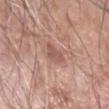Captured during whole-body skin photography for melanoma surveillance; the lesion was not biopsied.
A close-up tile cropped from a whole-body skin photograph, about 15 mm across.
A male patient about 60 years old.
On the right forearm.
An algorithmic analysis of the crop reported an average lesion color of about L≈55 a*≈23 b*≈26 (CIELAB) and a lesion–skin lightness drop of about 8.
Imaged with white-light lighting.